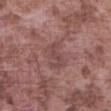follow-up = catalogued during a skin exam; not biopsied | acquisition = total-body-photography crop, ~15 mm field of view | lighting = white-light illumination | anatomic site = the abdomen | subject = male, approximately 75 years of age.